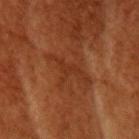Q: Who is the patient?
A: female, roughly 80 years of age
Q: Where on the body is the lesion?
A: the head or neck
Q: Lesion size?
A: about 5.5 mm
Q: Automated lesion metrics?
A: a lesion area of about 7 mm², a shape eccentricity near 0.9, and a shape-asymmetry score of about 0.5 (0 = symmetric); a border-irregularity index near 7.5/10, internal color variation of about 1.5 on a 0–10 scale, and radial color variation of about 0.5; a nevus-likeness score of about 0/100 and lesion-presence confidence of about 85/100
Q: What kind of image is this?
A: ~15 mm tile from a whole-body skin photo
Q: How was the tile lit?
A: cross-polarized